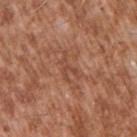From the right upper arm.
This is a white-light tile.
A male patient aged approximately 45.
A lesion tile, about 15 mm wide, cut from a 3D total-body photograph.
Longest diameter approximately 3 mm.
An algorithmic analysis of the crop reported a shape eccentricity near 0.8 and a symmetry-axis asymmetry near 0.65. And it measured an average lesion color of about L≈47 a*≈24 b*≈31 (CIELAB), a lesion–skin lightness drop of about 7, and a lesion-to-skin contrast of about 5 (normalized; higher = more distinct). It also reported a border-irregularity index near 9/10, internal color variation of about 0 on a 0–10 scale, and a peripheral color-asymmetry measure near 0. The analysis additionally found a detector confidence of about 85 out of 100 that the crop contains a lesion.